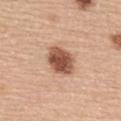{
  "patient": {
    "sex": "female",
    "age_approx": 65
  },
  "lighting": "white-light",
  "site": "upper back",
  "lesion_size": {
    "long_diameter_mm_approx": 4.0
  },
  "automated_metrics": {
    "cielab_L": 53,
    "cielab_a": 23,
    "cielab_b": 31,
    "border_irregularity_0_10": 1.5,
    "color_variation_0_10": 4.5,
    "peripheral_color_asymmetry": 1.5
  },
  "image": {
    "source": "total-body photography crop",
    "field_of_view_mm": 15
  }
}